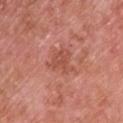workup: catalogued during a skin exam; not biopsied.